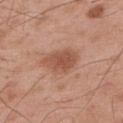Recorded during total-body skin imaging; not selected for excision or biopsy.
The tile uses white-light illumination.
The lesion is on the upper back.
The lesion's longest dimension is about 4.5 mm.
A male patient in their mid-50s.
Cropped from a total-body skin-imaging series; the visible field is about 15 mm.